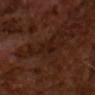• biopsy status: total-body-photography surveillance lesion; no biopsy
• automated lesion analysis: a symmetry-axis asymmetry near 0.4; a mean CIELAB color near L≈17 a*≈18 b*≈20 and a lesion–skin lightness drop of about 4; a color-variation rating of about 3.5/10 and a peripheral color-asymmetry measure near 1
• diameter: ≈3 mm
• illumination: cross-polarized illumination
• patient: male, aged 63 to 67
• image: 15 mm crop, total-body photography